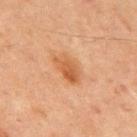notes: catalogued during a skin exam; not biopsied | site: the chest | image source: ~15 mm tile from a whole-body skin photo | illumination: cross-polarized | subject: male, aged 63 to 67 | lesion diameter: about 4 mm | automated metrics: roughly 8 lightness units darker than nearby skin and a normalized lesion–skin contrast near 7; a border-irregularity rating of about 2/10 and internal color variation of about 4.5 on a 0–10 scale; a nevus-likeness score of about 65/100.